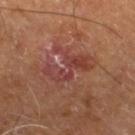Captured during whole-body skin photography for melanoma surveillance; the lesion was not biopsied. The lesion is on the leg. The subject is a male aged 58 to 62. Captured under cross-polarized illumination. A roughly 15 mm field-of-view crop from a total-body skin photograph.